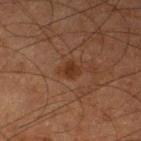The lesion is on the left lower leg.
A male subject about 65 years old.
This image is a 15 mm lesion crop taken from a total-body photograph.